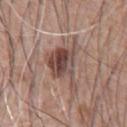The lesion was tiled from a total-body skin photograph and was not biopsied. A male subject in their 60s. On the chest. A 15 mm crop from a total-body photograph taken for skin-cancer surveillance.